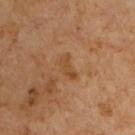lesion size: ~3 mm (longest diameter); patient: male, in their mid- to late 60s; acquisition: ~15 mm crop, total-body skin-cancer survey.